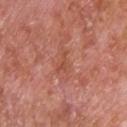Recorded during total-body skin imaging; not selected for excision or biopsy.
A lesion tile, about 15 mm wide, cut from a 3D total-body photograph.
A male subject in their mid- to late 60s.
The tile uses white-light illumination.
Located on the upper back.